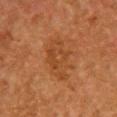Impression:
Recorded during total-body skin imaging; not selected for excision or biopsy.
Background:
Imaged with cross-polarized lighting. Longest diameter approximately 6 mm. An algorithmic analysis of the crop reported a footprint of about 15 mm² and two-axis asymmetry of about 0.25. A lesion tile, about 15 mm wide, cut from a 3D total-body photograph. A female patient, aged around 50. The lesion is located on the chest.